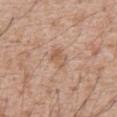Impression:
The lesion was tiled from a total-body skin photograph and was not biopsied.
Image and clinical context:
The subject is a male approximately 60 years of age. The lesion is on the abdomen. A 15 mm close-up extracted from a 3D total-body photography capture. About 2.5 mm across.